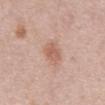Assessment:
No biopsy was performed on this lesion — it was imaged during a full skin examination and was not determined to be concerning.
Acquisition and patient details:
A 15 mm close-up tile from a total-body photography series done for melanoma screening. This is a white-light tile. Approximately 3.5 mm at its widest. Located on the abdomen. Automated image analysis of the tile measured a footprint of about 5.5 mm², an outline eccentricity of about 0.8 (0 = round, 1 = elongated), and a shape-asymmetry score of about 0.2 (0 = symmetric). And it measured an average lesion color of about L≈60 a*≈21 b*≈29 (CIELAB) and a normalized lesion–skin contrast near 6.5. It also reported a border-irregularity rating of about 2/10, a within-lesion color-variation index near 2.5/10, and a peripheral color-asymmetry measure near 1. A male subject in their 70s.